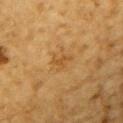Clinical impression: Imaged during a routine full-body skin examination; the lesion was not biopsied and no histopathology is available.